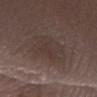{"biopsy_status": "not biopsied; imaged during a skin examination", "automated_metrics": {"border_irregularity_0_10": 3.5, "peripheral_color_asymmetry": 1.0, "nevus_likeness_0_100": 0, "lesion_detection_confidence_0_100": 85}, "image": {"source": "total-body photography crop", "field_of_view_mm": 15}, "lighting": "white-light", "site": "left forearm", "patient": {"sex": "female", "age_approx": 30}}